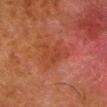Findings:
– follow-up — total-body-photography surveillance lesion; no biopsy
– size — about 4 mm
– lighting — cross-polarized
– patient — male, approximately 80 years of age
– image source — 15 mm crop, total-body photography
– location — the right lower leg